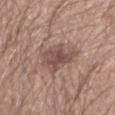Impression:
Recorded during total-body skin imaging; not selected for excision or biopsy.
Acquisition and patient details:
A male patient, aged 18–22. A 15 mm close-up tile from a total-body photography series done for melanoma screening. The lesion is on the left forearm. Imaged with white-light lighting.